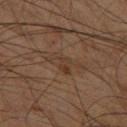This lesion was catalogued during total-body skin photography and was not selected for biopsy. The lesion's longest dimension is about 4 mm. Cropped from a whole-body photographic skin survey; the tile spans about 15 mm. Automated tile analysis of the lesion measured a lesion color around L≈32 a*≈14 b*≈24 in CIELAB and a lesion–skin lightness drop of about 5. The analysis additionally found a lesion-detection confidence of about 75/100. A male patient, roughly 60 years of age. On the right thigh. Captured under cross-polarized illumination.